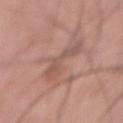{"biopsy_status": "not biopsied; imaged during a skin examination", "lesion_size": {"long_diameter_mm_approx": 4.5}, "image": {"source": "total-body photography crop", "field_of_view_mm": 15}, "site": "abdomen", "patient": {"sex": "male", "age_approx": 70}, "lighting": "white-light"}